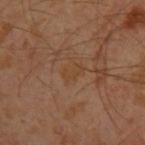<case>
  <patient>
    <sex>male</sex>
    <age_approx>30</age_approx>
  </patient>
  <site>arm</site>
  <image>
    <source>total-body photography crop</source>
    <field_of_view_mm>15</field_of_view_mm>
  </image>
  <lighting>cross-polarized</lighting>
  <lesion_size>
    <long_diameter_mm_approx>2.5</long_diameter_mm_approx>
  </lesion_size>
</case>